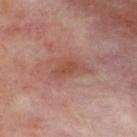Part of a total-body skin-imaging series; this lesion was reviewed on a skin check and was not flagged for biopsy.
The tile uses cross-polarized illumination.
Cropped from a total-body skin-imaging series; the visible field is about 15 mm.
The patient is a male approximately 50 years of age.
From the back.
The lesion's longest dimension is about 4.5 mm.
The total-body-photography lesion software estimated a mean CIELAB color near L≈48 a*≈24 b*≈27, roughly 8 lightness units darker than nearby skin, and a normalized lesion–skin contrast near 6.5. It also reported a border-irregularity index near 4.5/10 and internal color variation of about 1.5 on a 0–10 scale.